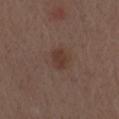TBP lesion metrics: a lesion color around L≈36 a*≈17 b*≈23 in CIELAB, about 7 CIELAB-L* units darker than the surrounding skin, and a normalized lesion–skin contrast near 6.5; a border-irregularity rating of about 2/10, a within-lesion color-variation index near 2/10, and a peripheral color-asymmetry measure near 0.5 | illumination: white-light | subject: male, aged 68 to 72 | body site: the arm | acquisition: total-body-photography crop, ~15 mm field of view.